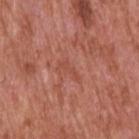Assessment:
Captured during whole-body skin photography for melanoma surveillance; the lesion was not biopsied.
Clinical summary:
Approximately 3 mm at its widest. A lesion tile, about 15 mm wide, cut from a 3D total-body photograph. The subject is a male roughly 65 years of age. The tile uses white-light illumination. Automated tile analysis of the lesion measured an eccentricity of roughly 0.95 and two-axis asymmetry of about 0.7. And it measured a lesion–skin lightness drop of about 6 and a lesion-to-skin contrast of about 4.5 (normalized; higher = more distinct). The analysis additionally found a border-irregularity index near 7.5/10, a color-variation rating of about 0/10, and radial color variation of about 0. The software also gave an automated nevus-likeness rating near 0 out of 100. The lesion is located on the back.